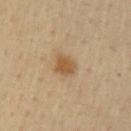{
  "biopsy_status": "not biopsied; imaged during a skin examination",
  "lighting": "cross-polarized",
  "patient": {
    "sex": "male",
    "age_approx": 65
  },
  "automated_metrics": {
    "area_mm2_approx": 5.0,
    "eccentricity": 0.45,
    "shape_asymmetry": 0.25,
    "border_irregularity_0_10": 2.0,
    "peripheral_color_asymmetry": 1.0,
    "nevus_likeness_0_100": 95,
    "lesion_detection_confidence_0_100": 100
  },
  "lesion_size": {
    "long_diameter_mm_approx": 2.5
  },
  "image": {
    "source": "total-body photography crop",
    "field_of_view_mm": 15
  },
  "site": "left upper arm"
}